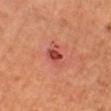This lesion was catalogued during total-body skin photography and was not selected for biopsy. The lesion's longest dimension is about 3.5 mm. A female patient, aged 63–67. From the leg. Captured under cross-polarized illumination. Automated tile analysis of the lesion measured an eccentricity of roughly 0.8 and two-axis asymmetry of about 0.25. And it measured an automated nevus-likeness rating near 30 out of 100. A roughly 15 mm field-of-view crop from a total-body skin photograph.